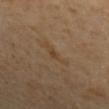This lesion was catalogued during total-body skin photography and was not selected for biopsy. Approximately 3.5 mm at its widest. Cropped from a whole-body photographic skin survey; the tile spans about 15 mm. The lesion-visualizer software estimated a mean CIELAB color near L≈43 a*≈15 b*≈31, a lesion–skin lightness drop of about 5, and a lesion-to-skin contrast of about 5 (normalized; higher = more distinct). The software also gave a peripheral color-asymmetry measure near 0. A male subject approximately 55 years of age. From the arm.